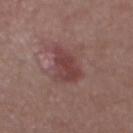Q: Was this lesion biopsied?
A: imaged on a skin check; not biopsied
Q: Who is the patient?
A: male, about 55 years old
Q: Where on the body is the lesion?
A: the abdomen
Q: How was this image acquired?
A: ~15 mm crop, total-body skin-cancer survey